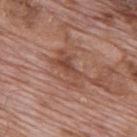The subject is a male roughly 70 years of age. Located on the mid back. A roughly 15 mm field-of-view crop from a total-body skin photograph. Captured under white-light illumination. Approximately 5 mm at its widest.